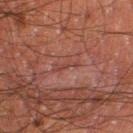Q: Automated lesion metrics?
A: an average lesion color of about L≈32 a*≈20 b*≈21 (CIELAB), about 5 CIELAB-L* units darker than the surrounding skin, and a normalized border contrast of about 5; a classifier nevus-likeness of about 0/100
Q: Where on the body is the lesion?
A: the right thigh
Q: Patient demographics?
A: male, roughly 50 years of age
Q: Lesion size?
A: ~2.5 mm (longest diameter)
Q: What kind of image is this?
A: ~15 mm crop, total-body skin-cancer survey
Q: What lighting was used for the tile?
A: cross-polarized illumination